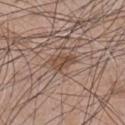Recorded during total-body skin imaging; not selected for excision or biopsy. A lesion tile, about 15 mm wide, cut from a 3D total-body photograph. The subject is a male in their mid-60s. On the chest. The lesion's longest dimension is about 3 mm. The total-body-photography lesion software estimated an area of roughly 4 mm² and an outline eccentricity of about 0.8 (0 = round, 1 = elongated). And it measured a lesion–skin lightness drop of about 9 and a lesion-to-skin contrast of about 7.5 (normalized; higher = more distinct). The software also gave border irregularity of about 3.5 on a 0–10 scale, internal color variation of about 3.5 on a 0–10 scale, and peripheral color asymmetry of about 1.5. Imaged with white-light lighting.